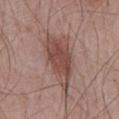Case summary:
– follow-up — imaged on a skin check; not biopsied
– image-analysis metrics — roughly 11 lightness units darker than nearby skin and a lesion-to-skin contrast of about 8 (normalized; higher = more distinct); a within-lesion color-variation index near 4/10; a lesion-detection confidence of about 100/100
– subject — male, aged approximately 70
– image — ~15 mm crop, total-body skin-cancer survey
– site — the abdomen
– tile lighting — white-light illumination
– lesion size — about 7.5 mm